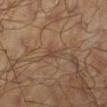biopsy_status: not biopsied; imaged during a skin examination
image:
  source: total-body photography crop
  field_of_view_mm: 15
lesion_size:
  long_diameter_mm_approx: 2.5
site: left lower leg
patient:
  sex: male
  age_approx: 45